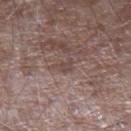The lesion was photographed on a routine skin check and not biopsied; there is no pathology result. Longest diameter approximately 2.5 mm. From the left forearm. A lesion tile, about 15 mm wide, cut from a 3D total-body photograph. The patient is a male aged 63–67. Captured under white-light illumination.